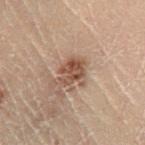A male subject about 70 years old. Imaged with cross-polarized lighting. The total-body-photography lesion software estimated a lesion color around L≈37 a*≈15 b*≈22 in CIELAB, about 9 CIELAB-L* units darker than the surrounding skin, and a normalized lesion–skin contrast near 8. It also reported a border-irregularity index near 3/10 and internal color variation of about 4 on a 0–10 scale. From the right lower leg. A close-up tile cropped from a whole-body skin photograph, about 15 mm across.